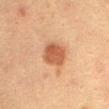Part of a total-body skin-imaging series; this lesion was reviewed on a skin check and was not flagged for biopsy. Automated tile analysis of the lesion measured an area of roughly 8 mm², an outline eccentricity of about 0.35 (0 = round, 1 = elongated), and a shape-asymmetry score of about 0.1 (0 = symmetric). And it measured a border-irregularity rating of about 1/10. Located on the abdomen. This image is a 15 mm lesion crop taken from a total-body photograph. The subject is a female aged 38–42. This is a cross-polarized tile.